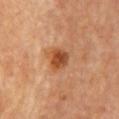{"biopsy_status": "not biopsied; imaged during a skin examination", "lighting": "cross-polarized", "lesion_size": {"long_diameter_mm_approx": 3.0}, "site": "chest", "patient": {"sex": "female", "age_approx": 70}, "image": {"source": "total-body photography crop", "field_of_view_mm": 15}, "automated_metrics": {"area_mm2_approx": 6.0, "eccentricity": 0.5, "color_variation_0_10": 4.5, "peripheral_color_asymmetry": 1.5, "nevus_likeness_0_100": 90, "lesion_detection_confidence_0_100": 100}}